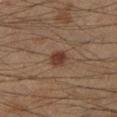biopsy status — catalogued during a skin exam; not biopsied
anatomic site — the left lower leg
patient — male, aged 38–42
image — total-body-photography crop, ~15 mm field of view